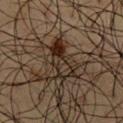Captured under cross-polarized illumination. Cropped from a whole-body photographic skin survey; the tile spans about 15 mm. The lesion's longest dimension is about 5 mm. The lesion is on the chest. A male subject aged approximately 60.